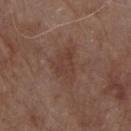Part of a total-body skin-imaging series; this lesion was reviewed on a skin check and was not flagged for biopsy.
This is a white-light tile.
Automated image analysis of the tile measured an automated nevus-likeness rating near 5 out of 100 and lesion-presence confidence of about 100/100.
The lesion's longest dimension is about 4.5 mm.
Cropped from a total-body skin-imaging series; the visible field is about 15 mm.
The patient is a male about 80 years old.
Located on the chest.